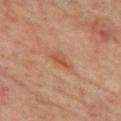The lesion was photographed on a routine skin check and not biopsied; there is no pathology result.
From the mid back.
This is a cross-polarized tile.
Longest diameter approximately 2.5 mm.
A male subject approximately 60 years of age.
Cropped from a total-body skin-imaging series; the visible field is about 15 mm.